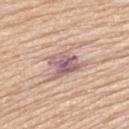Part of a total-body skin-imaging series; this lesion was reviewed on a skin check and was not flagged for biopsy. The lesion is on the upper back. The subject is a male in their mid-60s. Imaged with white-light lighting. About 4.5 mm across. A close-up tile cropped from a whole-body skin photograph, about 15 mm across.